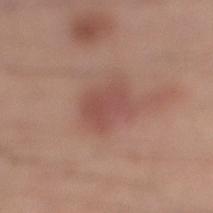Q: What is the lesion's diameter?
A: ~4 mm (longest diameter)
Q: What is the imaging modality?
A: ~15 mm crop, total-body skin-cancer survey
Q: Illumination type?
A: white-light illumination
Q: What are the patient's age and sex?
A: male, roughly 30 years of age
Q: Where on the body is the lesion?
A: the right lower leg